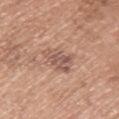Part of a total-body skin-imaging series; this lesion was reviewed on a skin check and was not flagged for biopsy. A lesion tile, about 15 mm wide, cut from a 3D total-body photograph. On the arm. The recorded lesion diameter is about 4 mm. Captured under white-light illumination. The subject is a male aged 73–77. An algorithmic analysis of the crop reported an area of roughly 8.5 mm² and an outline eccentricity of about 0.7 (0 = round, 1 = elongated).